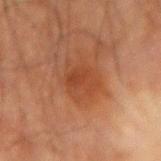Part of a total-body skin-imaging series; this lesion was reviewed on a skin check and was not flagged for biopsy. On the right forearm. A region of skin cropped from a whole-body photographic capture, roughly 15 mm wide. The tile uses cross-polarized illumination. The patient is a male about 65 years old.